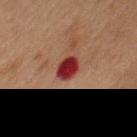| key | value |
|---|---|
| follow-up | imaged on a skin check; not biopsied |
| TBP lesion metrics | a border-irregularity rating of about 1/10, a color-variation rating of about 2/10, and radial color variation of about 0.5 |
| patient | male, aged around 50 |
| diameter | ≈3 mm |
| site | the chest |
| lighting | cross-polarized illumination |
| image source | total-body-photography crop, ~15 mm field of view |